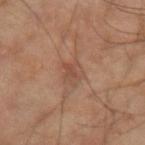{
  "biopsy_status": "not biopsied; imaged during a skin examination",
  "site": "right forearm",
  "image": {
    "source": "total-body photography crop",
    "field_of_view_mm": 15
  },
  "patient": {
    "sex": "male",
    "age_approx": 60
  },
  "lesion_size": {
    "long_diameter_mm_approx": 3.0
  },
  "lighting": "cross-polarized",
  "automated_metrics": {
    "area_mm2_approx": 4.5,
    "eccentricity": 0.7,
    "cielab_L": 42,
    "cielab_a": 18,
    "cielab_b": 26,
    "vs_skin_darker_L": 6.0,
    "border_irregularity_0_10": 4.0,
    "color_variation_0_10": 2.0
  }
}